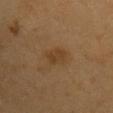Findings:
- notes — no biopsy performed (imaged during a skin exam)